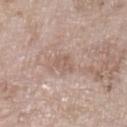Q: Was a biopsy performed?
A: imaged on a skin check; not biopsied
Q: Automated lesion metrics?
A: an outline eccentricity of about 0.7 (0 = round, 1 = elongated) and a symmetry-axis asymmetry near 0.35; a nevus-likeness score of about 0/100 and a lesion-detection confidence of about 100/100
Q: What is the lesion's diameter?
A: about 2.5 mm
Q: What lighting was used for the tile?
A: white-light
Q: What is the imaging modality?
A: 15 mm crop, total-body photography
Q: Who is the patient?
A: female, in their mid- to late 70s
Q: What is the anatomic site?
A: the left lower leg